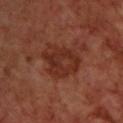Imaged during a routine full-body skin examination; the lesion was not biopsied and no histopathology is available. Automated image analysis of the tile measured a footprint of about 15 mm² and a shape-asymmetry score of about 0.25 (0 = symmetric). It also reported border irregularity of about 3 on a 0–10 scale and a peripheral color-asymmetry measure near 1.5. Located on the upper back. A close-up tile cropped from a whole-body skin photograph, about 15 mm across. A male subject approximately 70 years of age. About 5.5 mm across.